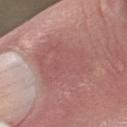biopsy status=catalogued during a skin exam; not biopsied
location=the left forearm
patient=male, approximately 75 years of age
image source=15 mm crop, total-body photography